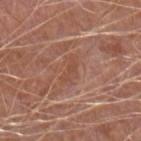workup: catalogued during a skin exam; not biopsied
image source: ~15 mm tile from a whole-body skin photo
subject: male, aged 63–67
site: the left forearm